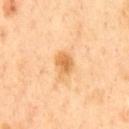The lesion was tiled from a total-body skin photograph and was not biopsied.
A male patient roughly 50 years of age.
On the chest.
A roughly 15 mm field-of-view crop from a total-body skin photograph.